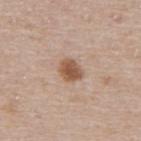Findings:
- notes: imaged on a skin check; not biopsied
- image source: ~15 mm tile from a whole-body skin photo
- lighting: white-light
- site: the upper back
- patient: female, aged 38 to 42
- size: ≈2.5 mm
- automated lesion analysis: two-axis asymmetry of about 0.25; a mean CIELAB color near L≈54 a*≈19 b*≈30 and roughly 13 lightness units darker than nearby skin